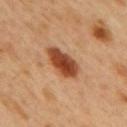follow-up: no biopsy performed (imaged during a skin exam)
subject: male, about 50 years old
site: the mid back
image source: ~15 mm crop, total-body skin-cancer survey
diameter: ≈5 mm